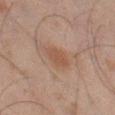<case>
  <biopsy_status>not biopsied; imaged during a skin examination</biopsy_status>
  <patient>
    <sex>male</sex>
    <age_approx>45</age_approx>
  </patient>
  <image>
    <source>total-body photography crop</source>
    <field_of_view_mm>15</field_of_view_mm>
  </image>
  <site>left thigh</site>
</case>